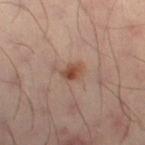Impression: Recorded during total-body skin imaging; not selected for excision or biopsy. Acquisition and patient details: Located on the left leg. The patient is a male in their 50s. Cropped from a total-body skin-imaging series; the visible field is about 15 mm.